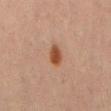The lesion was photographed on a routine skin check and not biopsied; there is no pathology result. Located on the front of the torso. Cropped from a whole-body photographic skin survey; the tile spans about 15 mm. Approximately 3 mm at its widest. The subject is a male about 70 years old. Captured under cross-polarized illumination.